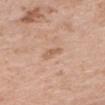Q: Was a biopsy performed?
A: total-body-photography surveillance lesion; no biopsy
Q: What are the patient's age and sex?
A: female, roughly 40 years of age
Q: What is the anatomic site?
A: the chest
Q: Illumination type?
A: white-light
Q: What is the imaging modality?
A: ~15 mm tile from a whole-body skin photo
Q: Lesion size?
A: ~2.5 mm (longest diameter)